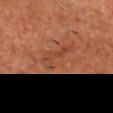follow-up=imaged on a skin check; not biopsied
image source=~15 mm tile from a whole-body skin photo
patient=male, aged around 80
lighting=cross-polarized illumination
body site=the chest
lesion size=≈5 mm